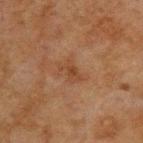Notes:
- biopsy status · total-body-photography surveillance lesion; no biopsy
- site · the upper back
- size · ≈3 mm
- illumination · cross-polarized illumination
- acquisition · ~15 mm crop, total-body skin-cancer survey
- subject · male, about 60 years old
- automated metrics · a lesion–skin lightness drop of about 5 and a normalized border contrast of about 6; a border-irregularity rating of about 4.5/10 and peripheral color asymmetry of about 0